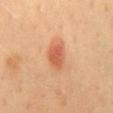follow-up: imaged on a skin check; not biopsied | diameter: ≈3.5 mm | patient: male, aged approximately 40 | site: the back | image: total-body-photography crop, ~15 mm field of view | lighting: cross-polarized illumination.